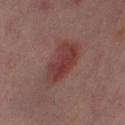Case summary:
* follow-up — total-body-photography surveillance lesion; no biopsy
* subject — female, about 50 years old
* imaging modality — total-body-photography crop, ~15 mm field of view
* site — the right thigh
* lesion size — about 5.5 mm